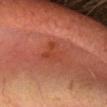Imaged during a routine full-body skin examination; the lesion was not biopsied and no histopathology is available. A female patient approximately 70 years of age. Captured under cross-polarized illumination. The lesion is on the head or neck. Approximately 3.5 mm at its widest. A 15 mm close-up tile from a total-body photography series done for melanoma screening.